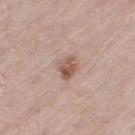The lesion was tiled from a total-body skin photograph and was not biopsied. A male subject, aged 58–62. Imaged with white-light lighting. A lesion tile, about 15 mm wide, cut from a 3D total-body photograph. Automated image analysis of the tile measured a lesion color around L≈55 a*≈20 b*≈26 in CIELAB, about 11 CIELAB-L* units darker than the surrounding skin, and a normalized border contrast of about 8. The lesion is located on the right thigh.